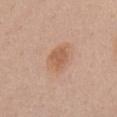notes: imaged on a skin check; not biopsied
image source: total-body-photography crop, ~15 mm field of view
patient: male, in their mid- to late 50s
body site: the chest